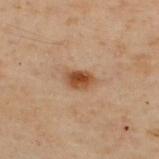Q: Is there a histopathology result?
A: imaged on a skin check; not biopsied
Q: Patient demographics?
A: male, roughly 50 years of age
Q: What is the lesion's diameter?
A: ~3 mm (longest diameter)
Q: Lesion location?
A: the back
Q: Illumination type?
A: cross-polarized illumination
Q: What kind of image is this?
A: total-body-photography crop, ~15 mm field of view